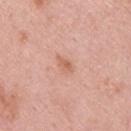Q: Is there a histopathology result?
A: no biopsy performed (imaged during a skin exam)
Q: What is the imaging modality?
A: ~15 mm tile from a whole-body skin photo
Q: What is the anatomic site?
A: the upper back
Q: What are the patient's age and sex?
A: male, aged 23 to 27
Q: What lighting was used for the tile?
A: white-light illumination
Q: Automated lesion metrics?
A: about 8 CIELAB-L* units darker than the surrounding skin